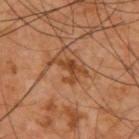{"site": "upper back", "image": {"source": "total-body photography crop", "field_of_view_mm": 15}, "lighting": "cross-polarized", "lesion_size": {"long_diameter_mm_approx": 4.5}, "patient": {"sex": "male", "age_approx": 60}}